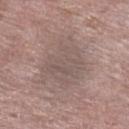On the left lower leg.
The patient is a female aged 68 to 72.
A roughly 15 mm field-of-view crop from a total-body skin photograph.